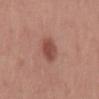Captured during whole-body skin photography for melanoma surveillance; the lesion was not biopsied. The lesion's longest dimension is about 3 mm. This is a white-light tile. A 15 mm close-up extracted from a 3D total-body photography capture. An algorithmic analysis of the crop reported a classifier nevus-likeness of about 95/100 and a detector confidence of about 100 out of 100 that the crop contains a lesion. The patient is a male aged 48 to 52. Located on the mid back.